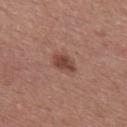Acquisition and patient details:
The subject is a female in their mid- to late 30s. On the upper back. Imaged with white-light lighting. Cropped from a whole-body photographic skin survey; the tile spans about 15 mm.